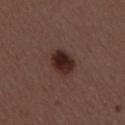Q: Is there a histopathology result?
A: imaged on a skin check; not biopsied
Q: What is the imaging modality?
A: total-body-photography crop, ~15 mm field of view
Q: How large is the lesion?
A: ~3.5 mm (longest diameter)
Q: Patient demographics?
A: female, aged 48–52
Q: What is the anatomic site?
A: the mid back
Q: How was the tile lit?
A: white-light